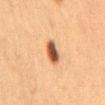Q: Was a biopsy performed?
A: total-body-photography surveillance lesion; no biopsy
Q: How was this image acquired?
A: total-body-photography crop, ~15 mm field of view
Q: What did automated image analysis measure?
A: two-axis asymmetry of about 0.25; border irregularity of about 2.5 on a 0–10 scale, a color-variation rating of about 6.5/10, and radial color variation of about 2; a classifier nevus-likeness of about 100/100 and a detector confidence of about 100 out of 100 that the crop contains a lesion
Q: What is the anatomic site?
A: the mid back
Q: What are the patient's age and sex?
A: female, roughly 60 years of age